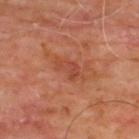workup=total-body-photography surveillance lesion; no biopsy
lighting=cross-polarized
image source=total-body-photography crop, ~15 mm field of view
subject=male, aged approximately 65
body site=the upper back